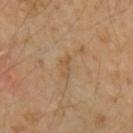{"image": {"source": "total-body photography crop", "field_of_view_mm": 15}, "automated_metrics": {"area_mm2_approx": 2.5, "eccentricity": 0.9, "shape_asymmetry": 0.45, "nevus_likeness_0_100": 0, "lesion_detection_confidence_0_100": 100}, "lesion_size": {"long_diameter_mm_approx": 3.0}, "patient": {"sex": "female", "age_approx": 45}, "lighting": "cross-polarized", "site": "left upper arm"}